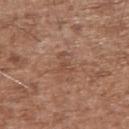<case>
  <biopsy_status>not biopsied; imaged during a skin examination</biopsy_status>
  <image>
    <source>total-body photography crop</source>
    <field_of_view_mm>15</field_of_view_mm>
  </image>
  <patient>
    <sex>male</sex>
    <age_approx>75</age_approx>
  </patient>
  <lesion_size>
    <long_diameter_mm_approx>2.5</long_diameter_mm_approx>
  </lesion_size>
  <site>arm</site>
</case>